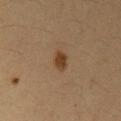This lesion was catalogued during total-body skin photography and was not selected for biopsy. The tile uses cross-polarized illumination. An algorithmic analysis of the crop reported a footprint of about 4 mm², a shape eccentricity near 0.75, and two-axis asymmetry of about 0.15. It also reported a lesion color around L≈36 a*≈18 b*≈30 in CIELAB, roughly 10 lightness units darker than nearby skin, and a normalized border contrast of about 9. A lesion tile, about 15 mm wide, cut from a 3D total-body photograph. From the arm. A female subject roughly 30 years of age. The lesion's longest dimension is about 2.5 mm.